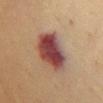No biopsy was performed on this lesion — it was imaged during a full skin examination and was not determined to be concerning.
This is a cross-polarized tile.
An algorithmic analysis of the crop reported an average lesion color of about L≈38 a*≈23 b*≈21 (CIELAB), a lesion–skin lightness drop of about 15, and a normalized lesion–skin contrast near 13.5. And it measured a border-irregularity index near 2/10, internal color variation of about 7 on a 0–10 scale, and radial color variation of about 2. It also reported an automated nevus-likeness rating near 75 out of 100.
The lesion is on the chest.
A region of skin cropped from a whole-body photographic capture, roughly 15 mm wide.
The subject is a female aged 53 to 57.
About 5.5 mm across.